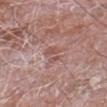This lesion was catalogued during total-body skin photography and was not selected for biopsy.
A male subject in their mid-70s.
Automated image analysis of the tile measured about 7 CIELAB-L* units darker than the surrounding skin and a normalized lesion–skin contrast near 5.5.
Cropped from a whole-body photographic skin survey; the tile spans about 15 mm.
Approximately 3 mm at its widest.
Located on the right forearm.
Captured under white-light illumination.